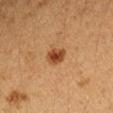The lesion was photographed on a routine skin check and not biopsied; there is no pathology result.
The total-body-photography lesion software estimated internal color variation of about 3 on a 0–10 scale and radial color variation of about 1. The software also gave an automated nevus-likeness rating near 100 out of 100 and a lesion-detection confidence of about 100/100.
Approximately 2.5 mm at its widest.
The tile uses cross-polarized illumination.
A female patient, in their 20s.
On the left upper arm.
A 15 mm close-up tile from a total-body photography series done for melanoma screening.